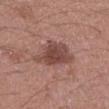workup: imaged on a skin check; not biopsied
subject: female, in their mid-50s
image-analysis metrics: a lesion area of about 13 mm² and an outline eccentricity of about 0.45 (0 = round, 1 = elongated); a border-irregularity rating of about 4/10, internal color variation of about 3.5 on a 0–10 scale, and a peripheral color-asymmetry measure near 1; an automated nevus-likeness rating near 65 out of 100
tile lighting: white-light
imaging modality: 15 mm crop, total-body photography
body site: the left forearm